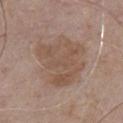Clinical impression: The lesion was photographed on a routine skin check and not biopsied; there is no pathology result. Acquisition and patient details: Located on the front of the torso. A male subject aged 73–77. A 15 mm close-up tile from a total-body photography series done for melanoma screening.